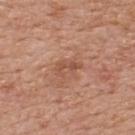biopsy status: total-body-photography surveillance lesion; no biopsy
subject: male, aged 58 to 62
lesion diameter: ~3 mm (longest diameter)
automated lesion analysis: two-axis asymmetry of about 0.4; a lesion-to-skin contrast of about 6 (normalized; higher = more distinct)
illumination: white-light illumination
imaging modality: ~15 mm crop, total-body skin-cancer survey
location: the upper back